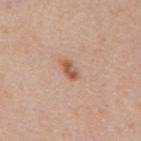Recorded during total-body skin imaging; not selected for excision or biopsy.
Imaged with white-light lighting.
Automated image analysis of the tile measured an average lesion color of about L≈56 a*≈21 b*≈31 (CIELAB), roughly 11 lightness units darker than nearby skin, and a normalized lesion–skin contrast near 8. It also reported a border-irregularity rating of about 2.5/10. And it measured an automated nevus-likeness rating near 80 out of 100 and a lesion-detection confidence of about 100/100.
A close-up tile cropped from a whole-body skin photograph, about 15 mm across.
Longest diameter approximately 3 mm.
A male patient, approximately 60 years of age.
On the back.